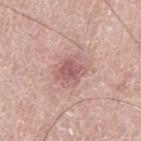No biopsy was performed on this lesion — it was imaged during a full skin examination and was not determined to be concerning.
The total-body-photography lesion software estimated a nevus-likeness score of about 5/100 and a lesion-detection confidence of about 100/100.
A male subject, about 75 years old.
Imaged with white-light lighting.
Cropped from a whole-body photographic skin survey; the tile spans about 15 mm.
Located on the left thigh.
Longest diameter approximately 4 mm.